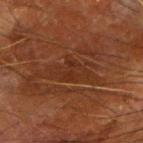notes = total-body-photography surveillance lesion; no biopsy | image = 15 mm crop, total-body photography | tile lighting = cross-polarized | subject = male, aged around 65 | location = the right forearm | lesion size = ~4.5 mm (longest diameter).